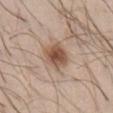The lesion was photographed on a routine skin check and not biopsied; there is no pathology result.
About 4 mm across.
From the chest.
Cropped from a whole-body photographic skin survey; the tile spans about 15 mm.
A male patient roughly 30 years of age.
Captured under white-light illumination.
The total-body-photography lesion software estimated a mean CIELAB color near L≈52 a*≈18 b*≈29, a lesion–skin lightness drop of about 13, and a normalized lesion–skin contrast near 9. And it measured border irregularity of about 2 on a 0–10 scale, a within-lesion color-variation index near 4.5/10, and peripheral color asymmetry of about 1. It also reported a nevus-likeness score of about 95/100 and a detector confidence of about 100 out of 100 that the crop contains a lesion.